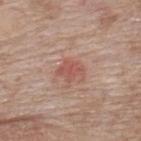  biopsy_status: not biopsied; imaged during a skin examination
  image:
    source: total-body photography crop
    field_of_view_mm: 15
  lesion_size:
    long_diameter_mm_approx: 2.5
  site: upper back
  patient:
    sex: female
    age_approx: 75